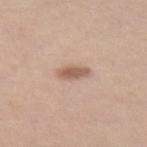The lesion was tiled from a total-body skin photograph and was not biopsied.
The tile uses white-light illumination.
A female subject, roughly 55 years of age.
This image is a 15 mm lesion crop taken from a total-body photograph.
The lesion-visualizer software estimated two-axis asymmetry of about 0.2. The software also gave a lesion color around L≈58 a*≈19 b*≈28 in CIELAB, about 12 CIELAB-L* units darker than the surrounding skin, and a normalized lesion–skin contrast near 8. And it measured a border-irregularity index near 2/10, a within-lesion color-variation index near 2.5/10, and peripheral color asymmetry of about 1. It also reported a nevus-likeness score of about 90/100 and a lesion-detection confidence of about 100/100.
About 3 mm across.
Located on the left thigh.